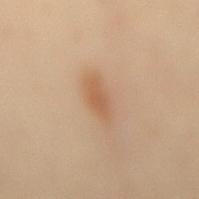Assessment: This lesion was catalogued during total-body skin photography and was not selected for biopsy. Background: The patient is a female in their 60s. The recorded lesion diameter is about 4.5 mm. The tile uses cross-polarized illumination. A close-up tile cropped from a whole-body skin photograph, about 15 mm across. The lesion is on the mid back.